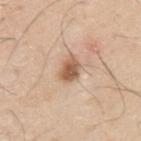- workup — catalogued during a skin exam; not biopsied
- image — ~15 mm tile from a whole-body skin photo
- TBP lesion metrics — an eccentricity of roughly 0.65
- patient — male, approximately 30 years of age
- body site — the arm
- lighting — white-light
- size — ~2.5 mm (longest diameter)